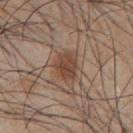No biopsy was performed on this lesion — it was imaged during a full skin examination and was not determined to be concerning.
From the chest.
An algorithmic analysis of the crop reported a footprint of about 9.5 mm², an eccentricity of roughly 0.7, and a symmetry-axis asymmetry near 0.3. And it measured about 9 CIELAB-L* units darker than the surrounding skin and a normalized border contrast of about 7.5. It also reported a border-irregularity rating of about 3/10, internal color variation of about 3 on a 0–10 scale, and a peripheral color-asymmetry measure near 1.
This is a white-light tile.
A male patient roughly 45 years of age.
A 15 mm close-up tile from a total-body photography series done for melanoma screening.
The lesion's longest dimension is about 4 mm.